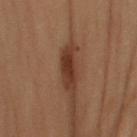Recorded during total-body skin imaging; not selected for excision or biopsy.
Located on the right forearm.
The subject is a male in their mid- to late 50s.
Measured at roughly 4 mm in maximum diameter.
A 15 mm close-up tile from a total-body photography series done for melanoma screening.